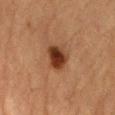lighting: cross-polarized illumination | location: the abdomen | image source: ~15 mm crop, total-body skin-cancer survey | patient: male, approximately 85 years of age or older | automated metrics: a border-irregularity index near 2.5/10, internal color variation of about 5 on a 0–10 scale, and peripheral color asymmetry of about 1.5.